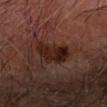Case summary:
• notes · no biopsy performed (imaged during a skin exam)
• image source · ~15 mm tile from a whole-body skin photo
• subject · male, aged 68 to 72
• location · the left forearm